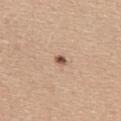Clinical impression: Imaged during a routine full-body skin examination; the lesion was not biopsied and no histopathology is available. Background: The patient is a female aged 33 to 37. Imaged with white-light lighting. The lesion's longest dimension is about 1.5 mm. A region of skin cropped from a whole-body photographic capture, roughly 15 mm wide. The lesion is located on the upper back.